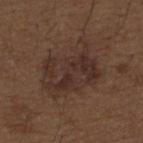patient: male, aged approximately 50
imaging modality: ~15 mm crop, total-body skin-cancer survey
illumination: white-light
body site: the back
size: ≈7.5 mm